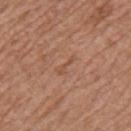Q: Was a biopsy performed?
A: catalogued during a skin exam; not biopsied
Q: How large is the lesion?
A: ≈2.5 mm
Q: Automated lesion metrics?
A: a border-irregularity rating of about 6/10, internal color variation of about 0 on a 0–10 scale, and radial color variation of about 0; a nevus-likeness score of about 0/100
Q: Patient demographics?
A: female, aged approximately 75
Q: What kind of image is this?
A: total-body-photography crop, ~15 mm field of view
Q: Where on the body is the lesion?
A: the left upper arm